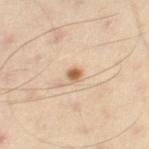Q: How was this image acquired?
A: 15 mm crop, total-body photography
Q: What did automated image analysis measure?
A: a border-irregularity rating of about 2/10 and radial color variation of about 0.5; a lesion-detection confidence of about 100/100
Q: How large is the lesion?
A: about 1.5 mm
Q: How was the tile lit?
A: cross-polarized
Q: Who is the patient?
A: male, about 55 years old
Q: Where on the body is the lesion?
A: the right thigh